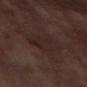Captured during whole-body skin photography for melanoma surveillance; the lesion was not biopsied.
A close-up tile cropped from a whole-body skin photograph, about 15 mm across.
The lesion's longest dimension is about 5 mm.
The total-body-photography lesion software estimated an area of roughly 12 mm², an eccentricity of roughly 0.75, and a symmetry-axis asymmetry near 0.25. It also reported lesion-presence confidence of about 75/100.
The tile uses cross-polarized illumination.
A female subject roughly 55 years of age.
The lesion is on the leg.